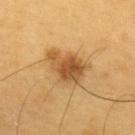The lesion was photographed on a routine skin check and not biopsied; there is no pathology result. The lesion is on the upper back. The subject is a male approximately 60 years of age. A 15 mm close-up tile from a total-body photography series done for melanoma screening.